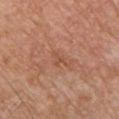lighting = white-light | subject = male, approximately 80 years of age | image = ~15 mm crop, total-body skin-cancer survey | anatomic site = the chest.